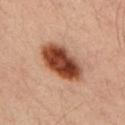notes: no biopsy performed (imaged during a skin exam)
image source: 15 mm crop, total-body photography
site: the right upper arm
subject: male, approximately 35 years of age